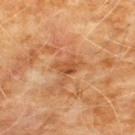notes: no biopsy performed (imaged during a skin exam); lighting: cross-polarized illumination; patient: male, aged around 60; anatomic site: the chest; TBP lesion metrics: a lesion area of about 4.5 mm², an eccentricity of roughly 0.7, and a symmetry-axis asymmetry near 0.4; acquisition: 15 mm crop, total-body photography; diameter: ≈3 mm.